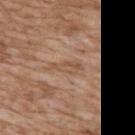workup: imaged on a skin check; not biopsied | illumination: white-light | imaging modality: 15 mm crop, total-body photography | anatomic site: the front of the torso | subject: male, about 60 years old.